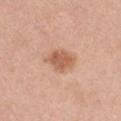image:
  source: total-body photography crop
  field_of_view_mm: 15
patient:
  sex: male
  age_approx: 60
lighting: white-light
site: left upper arm